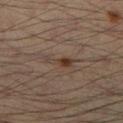{"biopsy_status": "not biopsied; imaged during a skin examination", "automated_metrics": {"area_mm2_approx": 3.5, "eccentricity": 0.95, "shape_asymmetry": 0.55, "border_irregularity_0_10": 6.5, "color_variation_0_10": 1.5}, "lesion_size": {"long_diameter_mm_approx": 4.0}, "image": {"source": "total-body photography crop", "field_of_view_mm": 15}, "patient": {"sex": "male", "age_approx": 35}, "site": "right lower leg", "lighting": "cross-polarized"}